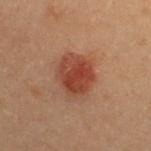workup — total-body-photography surveillance lesion; no biopsy | image source — ~15 mm tile from a whole-body skin photo | subject — female, aged 28 to 32 | site — the upper back.